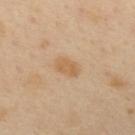- notes: no biopsy performed (imaged during a skin exam)
- lesion size: ~3 mm (longest diameter)
- illumination: cross-polarized
- anatomic site: the upper back
- automated lesion analysis: a lesion area of about 5 mm², an eccentricity of roughly 0.8, and a symmetry-axis asymmetry near 0.25; an average lesion color of about L≈61 a*≈17 b*≈38 (CIELAB), about 8 CIELAB-L* units darker than the surrounding skin, and a normalized lesion–skin contrast near 6.5; a color-variation rating of about 1.5/10 and radial color variation of about 0.5; a nevus-likeness score of about 15/100 and lesion-presence confidence of about 100/100
- subject: female, roughly 40 years of age
- image source: total-body-photography crop, ~15 mm field of view